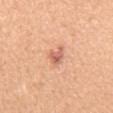Q: Was a biopsy performed?
A: no biopsy performed (imaged during a skin exam)
Q: How was this image acquired?
A: total-body-photography crop, ~15 mm field of view
Q: Who is the patient?
A: male, in their mid-50s
Q: What is the anatomic site?
A: the chest